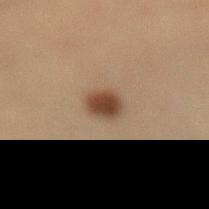workup: imaged on a skin check; not biopsied | subject: male, approximately 40 years of age | body site: the left lower leg | diameter: ~3 mm (longest diameter) | tile lighting: cross-polarized | image: total-body-photography crop, ~15 mm field of view.